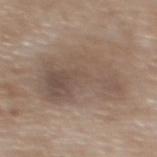Part of a total-body skin-imaging series; this lesion was reviewed on a skin check and was not flagged for biopsy. The patient is a female about 75 years old. The tile uses white-light illumination. A lesion tile, about 15 mm wide, cut from a 3D total-body photograph. An algorithmic analysis of the crop reported a lesion area of about 34 mm² and an eccentricity of roughly 0.85. The analysis additionally found a detector confidence of about 95 out of 100 that the crop contains a lesion. The lesion is located on the upper back.